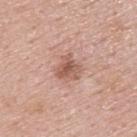Part of a total-body skin-imaging series; this lesion was reviewed on a skin check and was not flagged for biopsy.
A roughly 15 mm field-of-view crop from a total-body skin photograph.
The subject is a male aged 53–57.
Captured under white-light illumination.
The lesion is located on the upper back.